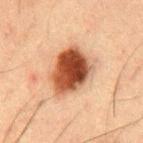A lesion tile, about 15 mm wide, cut from a 3D total-body photograph. The lesion's longest dimension is about 5.5 mm. The lesion is on the mid back. The subject is a male aged 48–52.